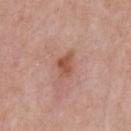Q: Is there a histopathology result?
A: no biopsy performed (imaged during a skin exam)
Q: Lesion size?
A: about 3 mm
Q: Illumination type?
A: white-light illumination
Q: What is the imaging modality?
A: ~15 mm tile from a whole-body skin photo
Q: Automated lesion metrics?
A: a lesion color around L≈52 a*≈24 b*≈30 in CIELAB, roughly 10 lightness units darker than nearby skin, and a normalized border contrast of about 8
Q: Who is the patient?
A: male, in their mid- to late 50s
Q: Lesion location?
A: the chest